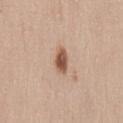Impression: Captured during whole-body skin photography for melanoma surveillance; the lesion was not biopsied. Context: A female subject, aged approximately 40. The lesion's longest dimension is about 3 mm. An algorithmic analysis of the crop reported an area of roughly 4.5 mm², an outline eccentricity of about 0.8 (0 = round, 1 = elongated), and two-axis asymmetry of about 0.3. It also reported a border-irregularity index near 2.5/10. A region of skin cropped from a whole-body photographic capture, roughly 15 mm wide. Located on the lower back. Imaged with white-light lighting.